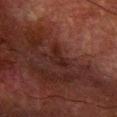This lesion was catalogued during total-body skin photography and was not selected for biopsy. From the right forearm. This is a cross-polarized tile. A roughly 15 mm field-of-view crop from a total-body skin photograph. The patient is a male aged 78 to 82.